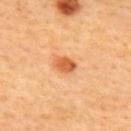image source: ~15 mm tile from a whole-body skin photo
subject: female, aged approximately 35
body site: the back